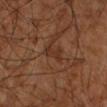The lesion was photographed on a routine skin check and not biopsied; there is no pathology result. Located on the right upper arm. A subject approximately 65 years of age. A 15 mm close-up extracted from a 3D total-body photography capture.